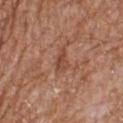biopsy status: imaged on a skin check; not biopsied | subject: male, aged 58–62 | lighting: white-light | anatomic site: the chest | diameter: about 2.5 mm | imaging modality: 15 mm crop, total-body photography.